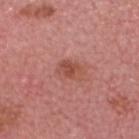Impression:
Captured during whole-body skin photography for melanoma surveillance; the lesion was not biopsied.
Image and clinical context:
A male patient in their mid-70s. The tile uses white-light illumination. A region of skin cropped from a whole-body photographic capture, roughly 15 mm wide. About 3 mm across. The lesion is located on the head or neck.